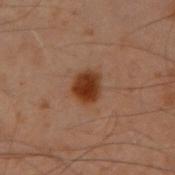workup: catalogued during a skin exam; not biopsied
image: ~15 mm crop, total-body skin-cancer survey
subject: male, roughly 50 years of age
location: the left arm